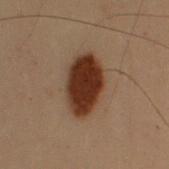{"biopsy_status": "not biopsied; imaged during a skin examination", "lesion_size": {"long_diameter_mm_approx": 6.0}, "lighting": "cross-polarized", "automated_metrics": {"cielab_L": 25, "cielab_a": 18, "cielab_b": 24, "vs_skin_darker_L": 15.0, "vs_skin_contrast_norm": 15.5, "border_irregularity_0_10": 1.5, "color_variation_0_10": 2.5, "peripheral_color_asymmetry": 1.0}, "image": {"source": "total-body photography crop", "field_of_view_mm": 15}, "site": "right upper arm", "patient": {"sex": "male", "age_approx": 55}}